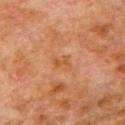Assessment:
Captured during whole-body skin photography for melanoma surveillance; the lesion was not biopsied.